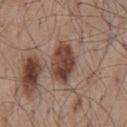Recorded during total-body skin imaging; not selected for excision or biopsy. Longest diameter approximately 5 mm. The lesion is on the mid back. This is a white-light tile. A male patient aged 53–57. A lesion tile, about 15 mm wide, cut from a 3D total-body photograph.